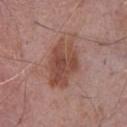Notes:
• follow-up · imaged on a skin check; not biopsied
• anatomic site · the chest
• patient · male, about 75 years old
• size · ≈6.5 mm
• acquisition · 15 mm crop, total-body photography
• lighting · white-light illumination